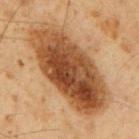Context:
The lesion is on the mid back. This image is a 15 mm lesion crop taken from a total-body photograph. A male subject, aged 58–62.